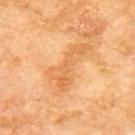The lesion was photographed on a routine skin check and not biopsied; there is no pathology result.
Located on the upper back.
The tile uses cross-polarized illumination.
The lesion's longest dimension is about 6 mm.
A region of skin cropped from a whole-body photographic capture, roughly 15 mm wide.
Automated tile analysis of the lesion measured a lesion area of about 9 mm² and a shape-asymmetry score of about 0.7 (0 = symmetric). And it measured a mean CIELAB color near L≈65 a*≈25 b*≈46, a lesion–skin lightness drop of about 8, and a normalized lesion–skin contrast near 5.5.
A male subject about 70 years old.